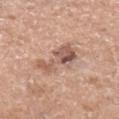Q: Was this lesion biopsied?
A: no biopsy performed (imaged during a skin exam)
Q: What are the patient's age and sex?
A: male, aged 63–67
Q: Lesion size?
A: about 4.5 mm
Q: How was the tile lit?
A: white-light
Q: What is the anatomic site?
A: the left forearm
Q: Automated lesion metrics?
A: an average lesion color of about L≈56 a*≈20 b*≈27 (CIELAB); a border-irregularity index near 7/10, a color-variation rating of about 8/10, and radial color variation of about 3; a nevus-likeness score of about 0/100 and a detector confidence of about 100 out of 100 that the crop contains a lesion
Q: How was this image acquired?
A: ~15 mm tile from a whole-body skin photo